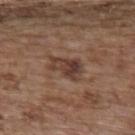Clinical summary: A 15 mm close-up extracted from a 3D total-body photography capture. Automated tile analysis of the lesion measured border irregularity of about 3.5 on a 0–10 scale and internal color variation of about 6.5 on a 0–10 scale. And it measured a detector confidence of about 100 out of 100 that the crop contains a lesion. The patient is a female approximately 65 years of age. Captured under white-light illumination. The lesion is on the upper back. The recorded lesion diameter is about 4 mm.